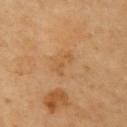This lesion was catalogued during total-body skin photography and was not selected for biopsy.
The recorded lesion diameter is about 3.5 mm.
A region of skin cropped from a whole-body photographic capture, roughly 15 mm wide.
Imaged with cross-polarized lighting.
A subject in their 60s.
Located on the left upper arm.